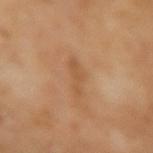| feature | finding |
|---|---|
| workup | no biopsy performed (imaged during a skin exam) |
| acquisition | ~15 mm tile from a whole-body skin photo |
| tile lighting | cross-polarized |
| subject | male, aged 63 to 67 |
| lesion size | ~4 mm (longest diameter) |